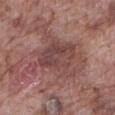Clinical impression:
Recorded during total-body skin imaging; not selected for excision or biopsy.
Image and clinical context:
Imaged with white-light lighting. About 7.5 mm across. The lesion is on the abdomen. The lesion-visualizer software estimated a footprint of about 27 mm², an eccentricity of roughly 0.6, and a shape-asymmetry score of about 0.45 (0 = symmetric). And it measured a lesion color around L≈45 a*≈22 b*≈21 in CIELAB, about 8 CIELAB-L* units darker than the surrounding skin, and a normalized lesion–skin contrast near 6.5. The analysis additionally found a color-variation rating of about 5/10 and a peripheral color-asymmetry measure near 1.5. This image is a 15 mm lesion crop taken from a total-body photograph. A male subject, aged 73–77.